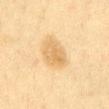No biopsy was performed on this lesion — it was imaged during a full skin examination and was not determined to be concerning.
A 15 mm crop from a total-body photograph taken for skin-cancer surveillance.
The lesion is on the abdomen.
A female patient, aged 38–42.
This is a cross-polarized tile.
The recorded lesion diameter is about 4 mm.